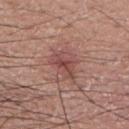workup = imaged on a skin check; not biopsied
imaging modality = total-body-photography crop, ~15 mm field of view
subject = male, in their 20s
size = ≈4 mm
location = the upper back
automated metrics = a mean CIELAB color near L≈48 a*≈24 b*≈23, a lesion–skin lightness drop of about 9, and a normalized lesion–skin contrast near 6.5; a nevus-likeness score of about 25/100 and lesion-presence confidence of about 100/100
lighting = white-light